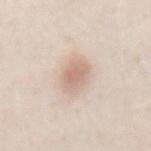Part of a total-body skin-imaging series; this lesion was reviewed on a skin check and was not flagged for biopsy.
The lesion is on the mid back.
Automated tile analysis of the lesion measured a mean CIELAB color near L≈68 a*≈17 b*≈26, about 11 CIELAB-L* units darker than the surrounding skin, and a lesion-to-skin contrast of about 6.5 (normalized; higher = more distinct). And it measured a border-irregularity index near 1.5/10 and radial color variation of about 1. The analysis additionally found an automated nevus-likeness rating near 95 out of 100 and a detector confidence of about 100 out of 100 that the crop contains a lesion.
A female patient, aged 23 to 27.
A 15 mm close-up tile from a total-body photography series done for melanoma screening.
About 4 mm across.